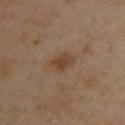Impression:
This lesion was catalogued during total-body skin photography and was not selected for biopsy.
Acquisition and patient details:
A close-up tile cropped from a whole-body skin photograph, about 15 mm across. From the upper back. A female subject aged 38–42. Imaged with cross-polarized lighting. The recorded lesion diameter is about 3 mm. An algorithmic analysis of the crop reported a footprint of about 4.5 mm², a shape eccentricity near 0.7, and a shape-asymmetry score of about 0.25 (0 = symmetric). And it measured a border-irregularity rating of about 2.5/10 and radial color variation of about 0.5.